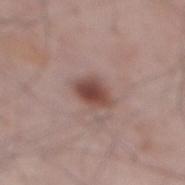This lesion was catalogued during total-body skin photography and was not selected for biopsy. The lesion is on the mid back. This is a white-light tile. A 15 mm crop from a total-body photograph taken for skin-cancer surveillance. The lesion-visualizer software estimated a symmetry-axis asymmetry near 0.2. The analysis additionally found border irregularity of about 2 on a 0–10 scale. And it measured an automated nevus-likeness rating near 95 out of 100 and a detector confidence of about 100 out of 100 that the crop contains a lesion. A male patient aged 63–67. Longest diameter approximately 4 mm.